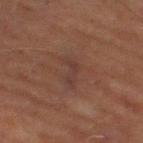Clinical impression:
No biopsy was performed on this lesion — it was imaged during a full skin examination and was not determined to be concerning.